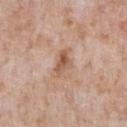notes = no biopsy performed (imaged during a skin exam).